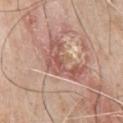Clinical impression:
No biopsy was performed on this lesion — it was imaged during a full skin examination and was not determined to be concerning.
Image and clinical context:
A male patient, aged around 80. A roughly 15 mm field-of-view crop from a total-body skin photograph. Measured at roughly 5.5 mm in maximum diameter. Located on the chest. The tile uses white-light illumination. The lesion-visualizer software estimated a within-lesion color-variation index near 4.5/10 and peripheral color asymmetry of about 1.